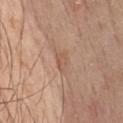Findings:
- TBP lesion metrics · a mean CIELAB color near L≈55 a*≈20 b*≈30, a lesion–skin lightness drop of about 7, and a lesion-to-skin contrast of about 5 (normalized; higher = more distinct); a border-irregularity index near 3.5/10, a within-lesion color-variation index near 2/10, and a peripheral color-asymmetry measure near 0.5; a classifier nevus-likeness of about 0/100
- anatomic site · the chest
- lighting · white-light
- patient · male, aged 58 to 62
- image · 15 mm crop, total-body photography